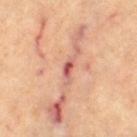Clinical impression: Captured during whole-body skin photography for melanoma surveillance; the lesion was not biopsied. Background: A female patient aged around 65. Automated image analysis of the tile measured an area of roughly 2.5 mm². The software also gave a border-irregularity rating of about 3/10, a within-lesion color-variation index near 0/10, and a peripheral color-asymmetry measure near 0. And it measured a classifier nevus-likeness of about 0/100. Approximately 3 mm at its widest. From the left thigh. A 15 mm close-up extracted from a 3D total-body photography capture. The tile uses cross-polarized illumination.